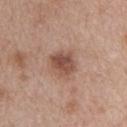Findings:
* workup · total-body-photography surveillance lesion; no biopsy